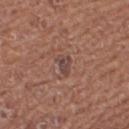Imaged during a routine full-body skin examination; the lesion was not biopsied and no histopathology is available.
The lesion is located on the right thigh.
The patient is a female aged approximately 50.
Cropped from a total-body skin-imaging series; the visible field is about 15 mm.
The total-body-photography lesion software estimated roughly 8 lightness units darker than nearby skin and a lesion-to-skin contrast of about 7 (normalized; higher = more distinct). The analysis additionally found a border-irregularity index near 4/10, a within-lesion color-variation index near 2.5/10, and peripheral color asymmetry of about 1. It also reported an automated nevus-likeness rating near 45 out of 100 and lesion-presence confidence of about 100/100.